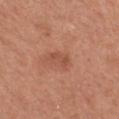  biopsy_status: not biopsied; imaged during a skin examination
  lighting: white-light
  site: chest
  lesion_size:
    long_diameter_mm_approx: 3.0
  patient:
    sex: female
    age_approx: 60
  automated_metrics:
    nevus_likeness_0_100: 0
  image:
    source: total-body photography crop
    field_of_view_mm: 15